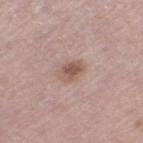Context: A female patient in their mid-60s. Imaged with white-light lighting. The total-body-photography lesion software estimated an area of roughly 5 mm², an eccentricity of roughly 0.8, and two-axis asymmetry of about 0.15. And it measured a border-irregularity index near 1.5/10 and radial color variation of about 1.5. The analysis additionally found an automated nevus-likeness rating near 60 out of 100. A roughly 15 mm field-of-view crop from a total-body skin photograph. Located on the left thigh. The recorded lesion diameter is about 3 mm.